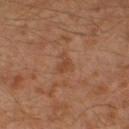Part of a total-body skin-imaging series; this lesion was reviewed on a skin check and was not flagged for biopsy. A 15 mm close-up tile from a total-body photography series done for melanoma screening. On the left leg. A male patient aged 28 to 32.